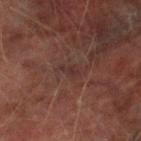The lesion is located on the right thigh. An algorithmic analysis of the crop reported an area of roughly 2 mm² and a symmetry-axis asymmetry near 0.6. And it measured a classifier nevus-likeness of about 0/100 and lesion-presence confidence of about 70/100. The recorded lesion diameter is about 2.5 mm. A roughly 15 mm field-of-view crop from a total-body skin photograph. This is a cross-polarized tile. A male subject about 75 years old.